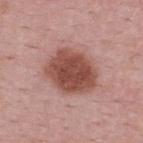Part of a total-body skin-imaging series; this lesion was reviewed on a skin check and was not flagged for biopsy. A male subject aged around 50. About 5.5 mm across. Imaged with white-light lighting. A 15 mm crop from a total-body photograph taken for skin-cancer surveillance. On the upper back.